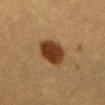workup — imaged on a skin check; not biopsied | lighting — cross-polarized illumination | diameter — about 4 mm | automated metrics — a footprint of about 11 mm², a shape eccentricity near 0.6, and a symmetry-axis asymmetry near 0.15; a lesion color around L≈30 a*≈18 b*≈29 in CIELAB and a normalized border contrast of about 12.5; peripheral color asymmetry of about 1 | imaging modality — 15 mm crop, total-body photography | subject — female, aged approximately 55 | anatomic site — the abdomen.